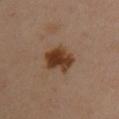Imaged during a routine full-body skin examination; the lesion was not biopsied and no histopathology is available.